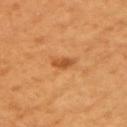{
  "biopsy_status": "not biopsied; imaged during a skin examination",
  "site": "arm",
  "lighting": "cross-polarized",
  "patient": {
    "sex": "female",
    "age_approx": 55
  },
  "image": {
    "source": "total-body photography crop",
    "field_of_view_mm": 15
  }
}